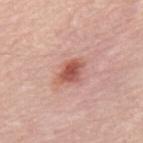Captured during whole-body skin photography for melanoma surveillance; the lesion was not biopsied.
A male subject, approximately 70 years of age.
A 15 mm close-up extracted from a 3D total-body photography capture.
This is a white-light tile.
On the mid back.